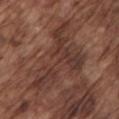follow-up: imaged on a skin check; not biopsied | tile lighting: white-light illumination | acquisition: ~15 mm crop, total-body skin-cancer survey | location: the left upper arm | image-analysis metrics: a footprint of about 27 mm², an outline eccentricity of about 0.8 (0 = round, 1 = elongated), and a shape-asymmetry score of about 0.7 (0 = symmetric); an average lesion color of about L≈36 a*≈19 b*≈23 (CIELAB), a lesion–skin lightness drop of about 8, and a normalized lesion–skin contrast near 7 | patient: male, about 75 years old.